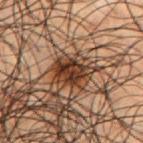workup — imaged on a skin check; not biopsied
patient — male, approximately 50 years of age
lighting — cross-polarized
lesion size — about 4 mm
acquisition — ~15 mm tile from a whole-body skin photo
body site — the chest